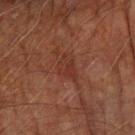<tbp_lesion>
  <biopsy_status>not biopsied; imaged during a skin examination</biopsy_status>
  <patient>
    <sex>male</sex>
    <age_approx>75</age_approx>
  </patient>
  <site>left forearm</site>
  <lighting>cross-polarized</lighting>
  <lesion_size>
    <long_diameter_mm_approx>3.5</long_diameter_mm_approx>
  </lesion_size>
  <image>
    <source>total-body photography crop</source>
    <field_of_view_mm>15</field_of_view_mm>
  </image>
  <automated_metrics>
    <cielab_L>27</cielab_L>
    <cielab_a>21</cielab_a>
    <cielab_b>23</cielab_b>
    <vs_skin_contrast_norm>5.5</vs_skin_contrast_norm>
    <nevus_likeness_0_100>0</nevus_likeness_0_100>
    <lesion_detection_confidence_0_100>95</lesion_detection_confidence_0_100>
  </automated_metrics>
</tbp_lesion>